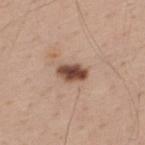Recorded during total-body skin imaging; not selected for excision or biopsy.